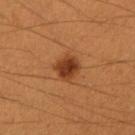{
  "biopsy_status": "not biopsied; imaged during a skin examination",
  "lesion_size": {
    "long_diameter_mm_approx": 3.0
  },
  "image": {
    "source": "total-body photography crop",
    "field_of_view_mm": 15
  },
  "site": "right forearm",
  "automated_metrics": {
    "cielab_L": 40,
    "cielab_a": 26,
    "cielab_b": 37,
    "vs_skin_contrast_norm": 10.0,
    "nevus_likeness_0_100": 100,
    "lesion_detection_confidence_0_100": 100
  },
  "patient": {
    "sex": "female",
    "age_approx": 40
  },
  "lighting": "cross-polarized"
}